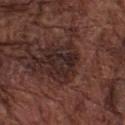Q: Was a biopsy performed?
A: catalogued during a skin exam; not biopsied
Q: What are the patient's age and sex?
A: male, about 75 years old
Q: How was this image acquired?
A: total-body-photography crop, ~15 mm field of view
Q: What is the anatomic site?
A: the front of the torso